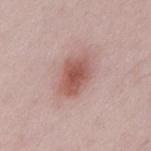notes=imaged on a skin check; not biopsied | acquisition=total-body-photography crop, ~15 mm field of view | patient=male, about 50 years old | diameter=≈4.5 mm | body site=the abdomen | lighting=white-light.